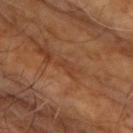No biopsy was performed on this lesion — it was imaged during a full skin examination and was not determined to be concerning.
About 2.5 mm across.
The tile uses cross-polarized illumination.
A male subject aged 58 to 62.
Located on the chest.
A region of skin cropped from a whole-body photographic capture, roughly 15 mm wide.